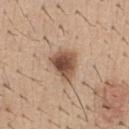Captured during whole-body skin photography for melanoma surveillance; the lesion was not biopsied. Located on the abdomen. A close-up tile cropped from a whole-body skin photograph, about 15 mm across. A male patient, approximately 40 years of age. The tile uses white-light illumination.